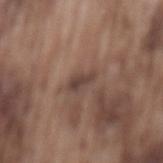A close-up tile cropped from a whole-body skin photograph, about 15 mm across.
The patient is a male in their mid- to late 70s.
The lesion is located on the mid back.